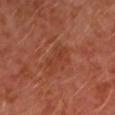Assessment: Part of a total-body skin-imaging series; this lesion was reviewed on a skin check and was not flagged for biopsy. Acquisition and patient details: The lesion's longest dimension is about 3.5 mm. A 15 mm crop from a total-body photograph taken for skin-cancer surveillance. The subject is a male about 30 years old. The lesion is on the left upper arm. The total-body-photography lesion software estimated an average lesion color of about L≈39 a*≈27 b*≈32 (CIELAB), roughly 5 lightness units darker than nearby skin, and a normalized border contrast of about 4.5. And it measured lesion-presence confidence of about 100/100.